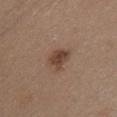<lesion>
  <biopsy_status>not biopsied; imaged during a skin examination</biopsy_status>
  <site>chest</site>
  <lesion_size>
    <long_diameter_mm_approx>3.0</long_diameter_mm_approx>
  </lesion_size>
  <patient>
    <sex>female</sex>
    <age_approx>45</age_approx>
  </patient>
  <lighting>white-light</lighting>
  <image>
    <source>total-body photography crop</source>
    <field_of_view_mm>15</field_of_view_mm>
  </image>
</lesion>